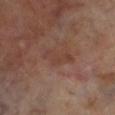Findings:
– biopsy status — imaged on a skin check; not biopsied
– patient — male, about 70 years old
– lesion diameter — ~4 mm (longest diameter)
– imaging modality — total-body-photography crop, ~15 mm field of view
– automated metrics — an average lesion color of about L≈39 a*≈19 b*≈24 (CIELAB), a lesion–skin lightness drop of about 5, and a normalized border contrast of about 5; a border-irregularity index near 5/10 and peripheral color asymmetry of about 0; a nevus-likeness score of about 0/100
– location — the left lower leg
– tile lighting — cross-polarized illumination